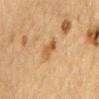Clinical impression:
Captured during whole-body skin photography for melanoma surveillance; the lesion was not biopsied.
Acquisition and patient details:
An algorithmic analysis of the crop reported a lesion area of about 4.5 mm². It also reported a lesion–skin lightness drop of about 9 and a normalized lesion–skin contrast near 7. The software also gave lesion-presence confidence of about 100/100. Imaged with cross-polarized lighting. A male subject, in their mid-80s or older. A region of skin cropped from a whole-body photographic capture, roughly 15 mm wide. Longest diameter approximately 3 mm. The lesion is located on the mid back.